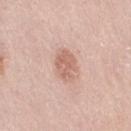From the right thigh. Longest diameter approximately 3.5 mm. A female subject, aged 63 to 67. The total-body-photography lesion software estimated a lesion area of about 7.5 mm², an eccentricity of roughly 0.7, and two-axis asymmetry of about 0.15. The analysis additionally found an average lesion color of about L≈63 a*≈21 b*≈28 (CIELAB) and about 10 CIELAB-L* units darker than the surrounding skin. A 15 mm crop from a total-body photograph taken for skin-cancer surveillance. Imaged with white-light lighting.